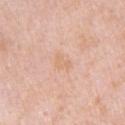The lesion was photographed on a routine skin check and not biopsied; there is no pathology result. Automated image analysis of the tile measured a footprint of about 3 mm² and a symmetry-axis asymmetry near 0.4. And it measured a border-irregularity index near 4/10, a within-lesion color-variation index near 0.5/10, and radial color variation of about 0. Approximately 2.5 mm at its widest. The tile uses white-light illumination. The subject is a female in their mid-40s. A 15 mm close-up tile from a total-body photography series done for melanoma screening. The lesion is on the left upper arm.